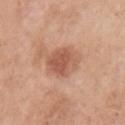- follow-up — no biopsy performed (imaged during a skin exam)
- lesion size — ~4.5 mm (longest diameter)
- patient — female, aged 53 to 57
- lighting — white-light illumination
- acquisition — 15 mm crop, total-body photography
- site — the left upper arm